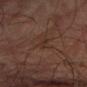<tbp_lesion>
<biopsy_status>not biopsied; imaged during a skin examination</biopsy_status>
<site>right upper arm</site>
<lesion_size>
  <long_diameter_mm_approx>2.5</long_diameter_mm_approx>
</lesion_size>
<lighting>cross-polarized</lighting>
<patient>
  <sex>male</sex>
  <age_approx>75</age_approx>
</patient>
<image>
  <source>total-body photography crop</source>
  <field_of_view_mm>15</field_of_view_mm>
</image>
</tbp_lesion>